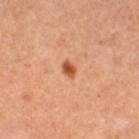Imaged during a routine full-body skin examination; the lesion was not biopsied and no histopathology is available. From the leg. Imaged with cross-polarized lighting. A female patient, roughly 30 years of age. A lesion tile, about 15 mm wide, cut from a 3D total-body photograph. Longest diameter approximately 2 mm.